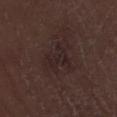Case summary:
– workup · total-body-photography surveillance lesion; no biopsy
– imaging modality · ~15 mm tile from a whole-body skin photo
– anatomic site · the leg
– subject · male, in their 70s